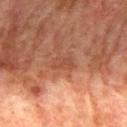<case>
<biopsy_status>not biopsied; imaged during a skin examination</biopsy_status>
<image>
  <source>total-body photography crop</source>
  <field_of_view_mm>15</field_of_view_mm>
</image>
<automated_metrics>
  <area_mm2_approx>4.0</area_mm2_approx>
  <vs_skin_darker_L>6.0</vs_skin_darker_L>
  <vs_skin_contrast_norm>5.0</vs_skin_contrast_norm>
  <border_irregularity_0_10>2.0</border_irregularity_0_10>
  <nevus_likeness_0_100>0</nevus_likeness_0_100>
</automated_metrics>
<patient>
  <sex>male</sex>
  <age_approx>75</age_approx>
</patient>
<lesion_size>
  <long_diameter_mm_approx>3.0</long_diameter_mm_approx>
</lesion_size>
<site>mid back</site>
<lighting>cross-polarized</lighting>
</case>